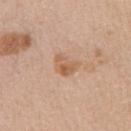Case summary:
- follow-up · catalogued during a skin exam; not biopsied